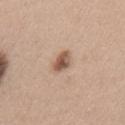follow-up = no biopsy performed (imaged during a skin exam).